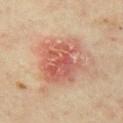Assessment: Imaged during a routine full-body skin examination; the lesion was not biopsied and no histopathology is available. Background: From the front of the torso. A female subject aged approximately 35. An algorithmic analysis of the crop reported a footprint of about 24 mm², an outline eccentricity of about 0.35 (0 = round, 1 = elongated), and two-axis asymmetry of about 0.2. The software also gave a mean CIELAB color near L≈56 a*≈26 b*≈30, roughly 10 lightness units darker than nearby skin, and a lesion-to-skin contrast of about 7 (normalized; higher = more distinct). And it measured border irregularity of about 3 on a 0–10 scale and a peripheral color-asymmetry measure near 2. This is a cross-polarized tile. A region of skin cropped from a whole-body photographic capture, roughly 15 mm wide.